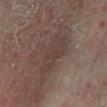Assessment:
The lesion was tiled from a total-body skin photograph and was not biopsied.
Background:
About 5.5 mm across. The total-body-photography lesion software estimated an automated nevus-likeness rating near 0 out of 100 and a lesion-detection confidence of about 65/100. The lesion is located on the leg. Cropped from a total-body skin-imaging series; the visible field is about 15 mm. This is a cross-polarized tile. A male patient, aged 68 to 72.